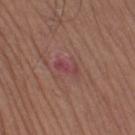Assessment: Part of a total-body skin-imaging series; this lesion was reviewed on a skin check and was not flagged for biopsy. Image and clinical context: On the right thigh. A region of skin cropped from a whole-body photographic capture, roughly 15 mm wide. Automated tile analysis of the lesion measured a lesion color around L≈43 a*≈27 b*≈19 in CIELAB, a lesion–skin lightness drop of about 6, and a lesion-to-skin contrast of about 6.5 (normalized; higher = more distinct). And it measured border irregularity of about 3 on a 0–10 scale. And it measured a nevus-likeness score of about 0/100 and a lesion-detection confidence of about 100/100. The subject is a male approximately 60 years of age.